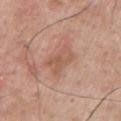Impression: The lesion was tiled from a total-body skin photograph and was not biopsied. Image and clinical context: A 15 mm close-up extracted from a 3D total-body photography capture. The lesion-visualizer software estimated a border-irregularity index near 3.5/10 and peripheral color asymmetry of about 1. A male subject about 60 years old. From the chest. The recorded lesion diameter is about 4 mm.